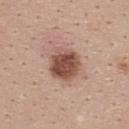| feature | finding |
|---|---|
| notes | catalogued during a skin exam; not biopsied |
| imaging modality | total-body-photography crop, ~15 mm field of view |
| tile lighting | white-light |
| image-analysis metrics | an area of roughly 12 mm², an outline eccentricity of about 0.15 (0 = round, 1 = elongated), and a symmetry-axis asymmetry near 0.1; an average lesion color of about L≈50 a*≈21 b*≈26 (CIELAB) |
| body site | the upper back |
| lesion size | ~4 mm (longest diameter) |
| subject | female, about 25 years old |